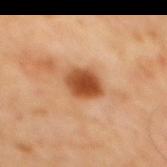<case>
  <biopsy_status>not biopsied; imaged during a skin examination</biopsy_status>
  <site>mid back</site>
  <patient>
    <sex>male</sex>
    <age_approx>65</age_approx>
  </patient>
  <image>
    <source>total-body photography crop</source>
    <field_of_view_mm>15</field_of_view_mm>
  </image>
  <lighting>cross-polarized</lighting>
</case>